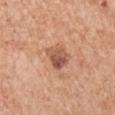Clinical impression:
Captured during whole-body skin photography for melanoma surveillance; the lesion was not biopsied.
Acquisition and patient details:
From the left upper arm. Imaged with white-light lighting. A 15 mm close-up extracted from a 3D total-body photography capture. The patient is a male approximately 65 years of age. An algorithmic analysis of the crop reported a lesion area of about 6 mm², a shape eccentricity near 0.6, and two-axis asymmetry of about 0.3.